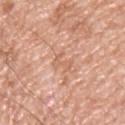Captured during whole-body skin photography for melanoma surveillance; the lesion was not biopsied.